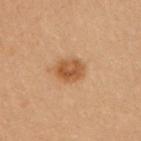This is a cross-polarized tile. A roughly 15 mm field-of-view crop from a total-body skin photograph. The total-body-photography lesion software estimated a lesion color around L≈43 a*≈19 b*≈32 in CIELAB and a lesion-to-skin contrast of about 8 (normalized; higher = more distinct). It also reported a border-irregularity index near 1/10, a within-lesion color-variation index near 3.5/10, and peripheral color asymmetry of about 1.5. The analysis additionally found an automated nevus-likeness rating near 95 out of 100 and lesion-presence confidence of about 100/100. The lesion is located on the left arm. A female subject approximately 30 years of age. Approximately 3 mm at its widest.